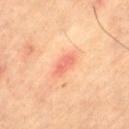Impression:
Part of a total-body skin-imaging series; this lesion was reviewed on a skin check and was not flagged for biopsy.